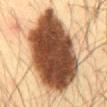  biopsy_status: not biopsied; imaged during a skin examination
  image:
    source: total-body photography crop
    field_of_view_mm: 15
  patient:
    sex: male
    age_approx: 35
  lighting: cross-polarized
  lesion_size:
    long_diameter_mm_approx: 12.0
  site: front of the torso